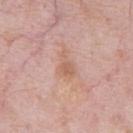Case summary:
- biopsy status · catalogued during a skin exam; not biopsied
- patient · male, in their 60s
- tile lighting · white-light
- body site · the abdomen
- image source · 15 mm crop, total-body photography
- image-analysis metrics · an eccentricity of roughly 0.8 and a symmetry-axis asymmetry near 0.45